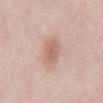  biopsy_status: not biopsied; imaged during a skin examination
  automated_metrics:
    cielab_L: 62
    cielab_a: 22
    cielab_b: 26
    vs_skin_darker_L: 9.0
    vs_skin_contrast_norm: 6.0
  patient:
    sex: male
    age_approx: 25
  lesion_size:
    long_diameter_mm_approx: 4.0
  site: abdomen
  image:
    source: total-body photography crop
    field_of_view_mm: 15